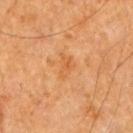{"biopsy_status": "not biopsied; imaged during a skin examination", "image": {"source": "total-body photography crop", "field_of_view_mm": 15}, "site": "right upper arm", "lighting": "cross-polarized", "automated_metrics": {"area_mm2_approx": 3.5, "shape_asymmetry": 0.55, "border_irregularity_0_10": 5.0, "peripheral_color_asymmetry": 0.5}, "patient": {"sex": "male", "age_approx": 60}, "lesion_size": {"long_diameter_mm_approx": 3.0}}